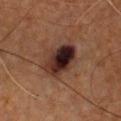The lesion was tiled from a total-body skin photograph and was not biopsied. This is a cross-polarized tile. From the front of the torso. The lesion's longest dimension is about 5 mm. Cropped from a total-body skin-imaging series; the visible field is about 15 mm. A male subject, approximately 65 years of age.